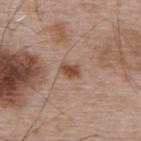Captured during whole-body skin photography for melanoma surveillance; the lesion was not biopsied.